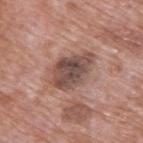notes = catalogued during a skin exam; not biopsied
body site = the back
acquisition = 15 mm crop, total-body photography
lighting = white-light
subject = male, in their 70s
lesion diameter = ≈5.5 mm
image-analysis metrics = a lesion area of about 14 mm²